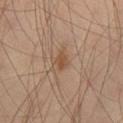notes = no biopsy performed (imaged during a skin exam) | patient = male, in their mid- to late 40s | imaging modality = ~15 mm crop, total-body skin-cancer survey | location = the right thigh | lesion size = ≈2.5 mm.